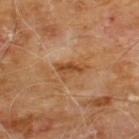biopsy status = no biopsy performed (imaged during a skin exam) | location = the chest | lighting = cross-polarized illumination | image = ~15 mm tile from a whole-body skin photo | patient = male, aged approximately 60 | lesion size = ~3.5 mm (longest diameter).